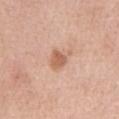  biopsy_status: not biopsied; imaged during a skin examination
  patient:
    sex: male
    age_approx: 75
  site: chest
  image:
    source: total-body photography crop
    field_of_view_mm: 15